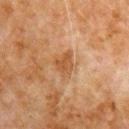| key | value |
|---|---|
| notes | imaged on a skin check; not biopsied |
| image-analysis metrics | a shape eccentricity near 0.65 and a symmetry-axis asymmetry near 0.35; a lesion color around L≈41 a*≈19 b*≈31 in CIELAB, roughly 7 lightness units darker than nearby skin, and a normalized border contrast of about 6.5; a border-irregularity index near 3.5/10, internal color variation of about 2.5 on a 0–10 scale, and radial color variation of about 1; a nevus-likeness score of about 0/100 and a lesion-detection confidence of about 100/100 |
| anatomic site | the right upper arm |
| illumination | cross-polarized illumination |
| image source | ~15 mm crop, total-body skin-cancer survey |
| patient | male, approximately 80 years of age |
| lesion size | ~3 mm (longest diameter) |